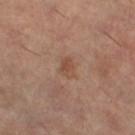No biopsy was performed on this lesion — it was imaged during a full skin examination and was not determined to be concerning. A female patient, approximately 60 years of age. The lesion's longest dimension is about 2.5 mm. Imaged with cross-polarized lighting. A 15 mm close-up extracted from a 3D total-body photography capture. The lesion-visualizer software estimated an area of roughly 3.5 mm², a shape eccentricity near 0.75, and a symmetry-axis asymmetry near 0.3. It also reported a border-irregularity index near 2.5/10 and peripheral color asymmetry of about 0.5. The software also gave an automated nevus-likeness rating near 5 out of 100 and a lesion-detection confidence of about 100/100. On the leg.